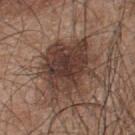Assessment: Recorded during total-body skin imaging; not selected for excision or biopsy. Image and clinical context: Captured under white-light illumination. The lesion is on the upper back. A roughly 15 mm field-of-view crop from a total-body skin photograph. The total-body-photography lesion software estimated an outline eccentricity of about 0.4 (0 = round, 1 = elongated). It also reported an average lesion color of about L≈38 a*≈17 b*≈23 (CIELAB), roughly 12 lightness units darker than nearby skin, and a normalized lesion–skin contrast near 10. It also reported a border-irregularity index near 4.5/10, a color-variation rating of about 5/10, and radial color variation of about 1.5. And it measured an automated nevus-likeness rating near 55 out of 100 and lesion-presence confidence of about 100/100. A male patient, aged approximately 45.